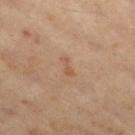Imaged during a routine full-body skin examination; the lesion was not biopsied and no histopathology is available.